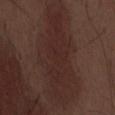<tbp_lesion>
<biopsy_status>not biopsied; imaged during a skin examination</biopsy_status>
<site>abdomen</site>
<patient>
  <sex>male</sex>
  <age_approx>70</age_approx>
</patient>
<image>
  <source>total-body photography crop</source>
  <field_of_view_mm>15</field_of_view_mm>
</image>
</tbp_lesion>